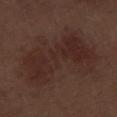{
  "biopsy_status": "not biopsied; imaged during a skin examination",
  "automated_metrics": {
    "cielab_L": 26,
    "cielab_a": 18,
    "cielab_b": 20,
    "vs_skin_darker_L": 6.0
  },
  "image": {
    "source": "total-body photography crop",
    "field_of_view_mm": 15
  },
  "site": "left thigh",
  "patient": {
    "sex": "male",
    "age_approx": 70
  },
  "lesion_size": {
    "long_diameter_mm_approx": 9.5
  },
  "lighting": "white-light"
}